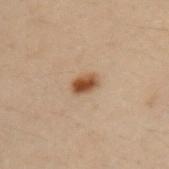This lesion was catalogued during total-body skin photography and was not selected for biopsy.
Imaged with cross-polarized lighting.
Cropped from a whole-body photographic skin survey; the tile spans about 15 mm.
Located on the arm.
An algorithmic analysis of the crop reported an area of roughly 4 mm² and two-axis asymmetry of about 0.2. The software also gave border irregularity of about 2 on a 0–10 scale, a color-variation rating of about 4/10, and peripheral color asymmetry of about 1. And it measured a classifier nevus-likeness of about 100/100 and lesion-presence confidence of about 100/100.
The patient is a male aged 28–32.